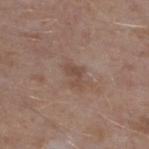The lesion was tiled from a total-body skin photograph and was not biopsied.
The lesion's longest dimension is about 3 mm.
The tile uses white-light illumination.
The lesion is on the left lower leg.
Cropped from a whole-body photographic skin survey; the tile spans about 15 mm.
Automated tile analysis of the lesion measured a shape eccentricity near 0.8 and two-axis asymmetry of about 0.45. And it measured a lesion color around L≈47 a*≈18 b*≈24 in CIELAB, roughly 7 lightness units darker than nearby skin, and a normalized border contrast of about 5.5. It also reported a nevus-likeness score of about 0/100 and lesion-presence confidence of about 100/100.
A male patient, aged approximately 45.